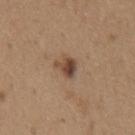Clinical impression:
Recorded during total-body skin imaging; not selected for excision or biopsy.
Image and clinical context:
The tile uses white-light illumination. A male subject, aged 63–67. The recorded lesion diameter is about 3 mm. Automated tile analysis of the lesion measured an area of roughly 4.5 mm², an outline eccentricity of about 0.6 (0 = round, 1 = elongated), and a symmetry-axis asymmetry near 0.35. And it measured a lesion color around L≈45 a*≈18 b*≈28 in CIELAB. The software also gave a border-irregularity index near 3.5/10 and peripheral color asymmetry of about 1.5. Located on the chest. A 15 mm close-up tile from a total-body photography series done for melanoma screening.